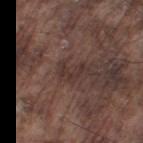{
  "biopsy_status": "not biopsied; imaged during a skin examination",
  "lighting": "white-light",
  "lesion_size": {
    "long_diameter_mm_approx": 2.5
  },
  "image": {
    "source": "total-body photography crop",
    "field_of_view_mm": 15
  },
  "site": "leg",
  "patient": {
    "sex": "male",
    "age_approx": 75
  }
}